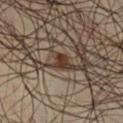Assessment:
The lesion was tiled from a total-body skin photograph and was not biopsied.
Acquisition and patient details:
Captured under cross-polarized illumination. Measured at roughly 2.5 mm in maximum diameter. A region of skin cropped from a whole-body photographic capture, roughly 15 mm wide. From the left thigh. The patient is a male aged approximately 50.